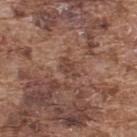Q: Is there a histopathology result?
A: catalogued during a skin exam; not biopsied
Q: Where on the body is the lesion?
A: the back
Q: What is the lesion's diameter?
A: about 2.5 mm
Q: What are the patient's age and sex?
A: male, approximately 75 years of age
Q: What kind of image is this?
A: total-body-photography crop, ~15 mm field of view